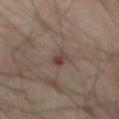Case summary:
• biopsy status: catalogued during a skin exam; not biopsied
• location: the abdomen
• size: ≈2.5 mm
• automated lesion analysis: a lesion area of about 2.5 mm², an outline eccentricity of about 0.9 (0 = round, 1 = elongated), and two-axis asymmetry of about 0.3; a border-irregularity index near 3/10 and radial color variation of about 0
• image: ~15 mm crop, total-body skin-cancer survey
• subject: male, approximately 65 years of age
• tile lighting: cross-polarized